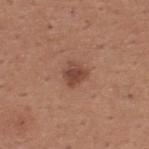Q: Is there a histopathology result?
A: imaged on a skin check; not biopsied
Q: Automated lesion metrics?
A: a footprint of about 5 mm², an outline eccentricity of about 0.4 (0 = round, 1 = elongated), and a shape-asymmetry score of about 0.25 (0 = symmetric); a lesion color around L≈45 a*≈22 b*≈28 in CIELAB, about 10 CIELAB-L* units darker than the surrounding skin, and a normalized border contrast of about 7.5; a classifier nevus-likeness of about 80/100 and lesion-presence confidence of about 100/100
Q: What kind of image is this?
A: ~15 mm tile from a whole-body skin photo
Q: Patient demographics?
A: male, aged 63–67
Q: What is the anatomic site?
A: the upper back
Q: What lighting was used for the tile?
A: white-light